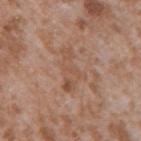| feature | finding |
|---|---|
| workup | no biopsy performed (imaged during a skin exam) |
| TBP lesion metrics | a footprint of about 7.5 mm², an eccentricity of roughly 0.9, and a shape-asymmetry score of about 0.45 (0 = symmetric); internal color variation of about 3.5 on a 0–10 scale and radial color variation of about 1; an automated nevus-likeness rating near 0 out of 100 |
| imaging modality | ~15 mm crop, total-body skin-cancer survey |
| patient | male, aged 43–47 |
| lighting | white-light illumination |
| anatomic site | the arm |
| size | ~5 mm (longest diameter) |